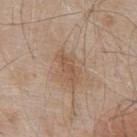Assessment: The lesion was tiled from a total-body skin photograph and was not biopsied. Image and clinical context: The patient is a male aged 53–57. The lesion is located on the upper back. A roughly 15 mm field-of-view crop from a total-body skin photograph.